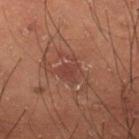A 15 mm crop from a total-body photograph taken for skin-cancer surveillance. The lesion is on the back. A male patient, roughly 50 years of age.